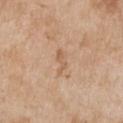The lesion was tiled from a total-body skin photograph and was not biopsied. A female patient, aged 63 to 67. A 15 mm close-up extracted from a 3D total-body photography capture. Imaged with white-light lighting. About 3.5 mm across. The lesion is located on the chest.